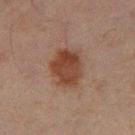Impression: The lesion was photographed on a routine skin check and not biopsied; there is no pathology result. Image and clinical context: On the leg. Imaged with cross-polarized lighting. A region of skin cropped from a whole-body photographic capture, roughly 15 mm wide. Measured at roughly 4.5 mm in maximum diameter. A male patient, aged around 45. The total-body-photography lesion software estimated an average lesion color of about L≈34 a*≈18 b*≈24 (CIELAB), about 9 CIELAB-L* units darker than the surrounding skin, and a lesion-to-skin contrast of about 9 (normalized; higher = more distinct). And it measured a classifier nevus-likeness of about 95/100 and a lesion-detection confidence of about 100/100.